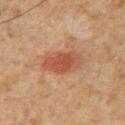Q: Is there a histopathology result?
A: imaged on a skin check; not biopsied
Q: What kind of image is this?
A: total-body-photography crop, ~15 mm field of view
Q: Illumination type?
A: cross-polarized
Q: Lesion size?
A: about 4.5 mm
Q: Who is the patient?
A: male, aged around 50
Q: Where on the body is the lesion?
A: the chest